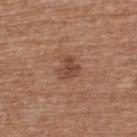{"biopsy_status": "not biopsied; imaged during a skin examination", "lighting": "white-light", "automated_metrics": {"area_mm2_approx": 5.0, "shape_asymmetry": 0.4, "border_irregularity_0_10": 4.0, "color_variation_0_10": 2.5, "peripheral_color_asymmetry": 1.0}, "lesion_size": {"long_diameter_mm_approx": 3.0}, "site": "back", "patient": {"sex": "female", "age_approx": 75}, "image": {"source": "total-body photography crop", "field_of_view_mm": 15}}